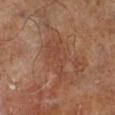Captured during whole-body skin photography for melanoma surveillance; the lesion was not biopsied. This is a cross-polarized tile. The lesion's longest dimension is about 6.5 mm. The subject is a male aged 68 to 72. Automated tile analysis of the lesion measured a footprint of about 15 mm², a shape eccentricity near 0.85, and two-axis asymmetry of about 0.35. The analysis additionally found an average lesion color of about L≈35 a*≈18 b*≈25 (CIELAB), roughly 5 lightness units darker than nearby skin, and a lesion-to-skin contrast of about 5 (normalized; higher = more distinct). From the right lower leg. A 15 mm crop from a total-body photograph taken for skin-cancer surveillance.